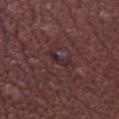No biopsy was performed on this lesion — it was imaged during a full skin examination and was not determined to be concerning. On the left thigh. This is a white-light tile. The lesion's longest dimension is about 3 mm. A male patient roughly 55 years of age. A region of skin cropped from a whole-body photographic capture, roughly 15 mm wide.